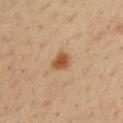Clinical impression: Part of a total-body skin-imaging series; this lesion was reviewed on a skin check and was not flagged for biopsy. Image and clinical context: This is a cross-polarized tile. A male patient approximately 35 years of age. Cropped from a total-body skin-imaging series; the visible field is about 15 mm. The lesion is on the right upper arm.